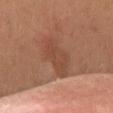Imaged during a routine full-body skin examination; the lesion was not biopsied and no histopathology is available.
Located on the abdomen.
A male patient aged around 55.
A region of skin cropped from a whole-body photographic capture, roughly 15 mm wide.
Imaged with cross-polarized lighting.
Approximately 6 mm at its widest.